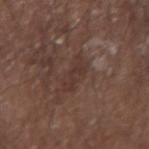This is a white-light tile. The lesion is on the arm. This image is a 15 mm lesion crop taken from a total-body photograph. A male subject aged 63 to 67. Approximately 3.5 mm at its widest. Automated tile analysis of the lesion measured a lesion area of about 4 mm², a shape eccentricity near 0.9, and a shape-asymmetry score of about 0.55 (0 = symmetric). The analysis additionally found a lesion–skin lightness drop of about 6 and a normalized border contrast of about 6. It also reported a border-irregularity index near 8.5/10, a color-variation rating of about 0/10, and a peripheral color-asymmetry measure near 0.